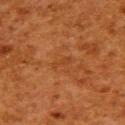The subject is a female roughly 50 years of age.
Cropped from a whole-body photographic skin survey; the tile spans about 15 mm.
The lesion is located on the upper back.
About 2.5 mm across.